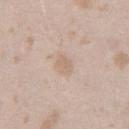Located on the right thigh.
The tile uses white-light illumination.
Measured at roughly 2.5 mm in maximum diameter.
Cropped from a whole-body photographic skin survey; the tile spans about 15 mm.
A female patient, aged 23 to 27.
The total-body-photography lesion software estimated an area of roughly 4 mm², a shape eccentricity near 0.7, and a shape-asymmetry score of about 0.15 (0 = symmetric). The software also gave a nevus-likeness score of about 0/100 and lesion-presence confidence of about 100/100.